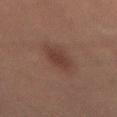Q: Was a biopsy performed?
A: catalogued during a skin exam; not biopsied
Q: What is the anatomic site?
A: the mid back
Q: How large is the lesion?
A: ~4.5 mm (longest diameter)
Q: What are the patient's age and sex?
A: male, roughly 45 years of age
Q: How was this image acquired?
A: 15 mm crop, total-body photography
Q: Automated lesion metrics?
A: a lesion area of about 9 mm², an outline eccentricity of about 0.85 (0 = round, 1 = elongated), and two-axis asymmetry of about 0.25